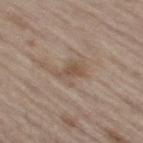Q: Illumination type?
A: white-light
Q: How large is the lesion?
A: about 3 mm
Q: What is the anatomic site?
A: the right thigh
Q: What kind of image is this?
A: ~15 mm tile from a whole-body skin photo
Q: What are the patient's age and sex?
A: male, about 70 years old